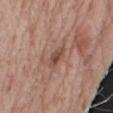Recorded during total-body skin imaging; not selected for excision or biopsy. A male subject, approximately 75 years of age. An algorithmic analysis of the crop reported a mean CIELAB color near L≈47 a*≈21 b*≈27, roughly 10 lightness units darker than nearby skin, and a normalized lesion–skin contrast near 7.5. The analysis additionally found a classifier nevus-likeness of about 0/100 and a detector confidence of about 100 out of 100 that the crop contains a lesion. From the right upper arm. The tile uses white-light illumination. A 15 mm crop from a total-body photograph taken for skin-cancer surveillance. About 2.5 mm across.